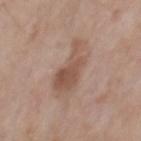Impression: Part of a total-body skin-imaging series; this lesion was reviewed on a skin check and was not flagged for biopsy. Context: The lesion-visualizer software estimated a border-irregularity index near 5.5/10, a color-variation rating of about 3.5/10, and peripheral color asymmetry of about 1.5. It also reported an automated nevus-likeness rating near 55 out of 100 and lesion-presence confidence of about 100/100. This is a white-light tile. A male subject, in their 80s. Longest diameter approximately 5 mm. A close-up tile cropped from a whole-body skin photograph, about 15 mm across. From the chest.